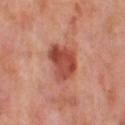imaging modality: total-body-photography crop, ~15 mm field of view | location: the right thigh | subject: female, about 50 years old | tile lighting: cross-polarized | diameter: ≈4.5 mm.